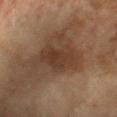{"biopsy_status": "not biopsied; imaged during a skin examination", "image": {"source": "total-body photography crop", "field_of_view_mm": 15}, "lighting": "cross-polarized", "lesion_size": {"long_diameter_mm_approx": 4.0}, "patient": {"sex": "female", "age_approx": 70}, "site": "left forearm"}